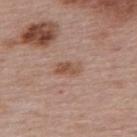This lesion was catalogued during total-body skin photography and was not selected for biopsy.
The lesion is located on the upper back.
The recorded lesion diameter is about 3 mm.
A close-up tile cropped from a whole-body skin photograph, about 15 mm across.
The patient is a female aged approximately 50.